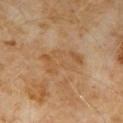Case summary:
– workup — no biopsy performed (imaged during a skin exam)
– subject — male, roughly 60 years of age
– location — the chest
– lesion size — ~5 mm (longest diameter)
– image — 15 mm crop, total-body photography
– automated lesion analysis — an area of roughly 15 mm², a shape eccentricity near 0.75, and two-axis asymmetry of about 0.25